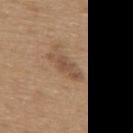Clinical impression:
This lesion was catalogued during total-body skin photography and was not selected for biopsy.
Background:
On the upper back. Longest diameter approximately 4.5 mm. A 15 mm crop from a total-body photograph taken for skin-cancer surveillance. Captured under white-light illumination. A male subject aged approximately 65.